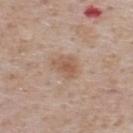biopsy status: total-body-photography surveillance lesion; no biopsy
body site: the back
automated lesion analysis: a footprint of about 5.5 mm², a shape eccentricity near 0.65, and two-axis asymmetry of about 0.3; lesion-presence confidence of about 100/100
subject: male, aged 73 to 77
image: ~15 mm tile from a whole-body skin photo